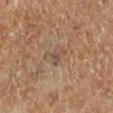{
  "biopsy_status": "not biopsied; imaged during a skin examination",
  "site": "right lower leg",
  "patient": {
    "sex": "female"
  },
  "image": {
    "source": "total-body photography crop",
    "field_of_view_mm": 15
  }
}